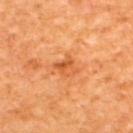The lesion was tiled from a total-body skin photograph and was not biopsied. A 15 mm crop from a total-body photograph taken for skin-cancer surveillance. The subject is a male about 65 years old. On the upper back.